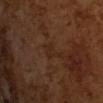Part of a total-body skin-imaging series; this lesion was reviewed on a skin check and was not flagged for biopsy.
Measured at roughly 3.5 mm in maximum diameter.
Imaged with cross-polarized lighting.
A 15 mm close-up extracted from a 3D total-body photography capture.
The subject is a male aged 63–67.